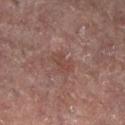Part of a total-body skin-imaging series; this lesion was reviewed on a skin check and was not flagged for biopsy. Located on the right leg. Imaged with cross-polarized lighting. The lesion-visualizer software estimated a classifier nevus-likeness of about 0/100. A 15 mm crop from a total-body photograph taken for skin-cancer surveillance. The patient is a female roughly 80 years of age. Measured at roughly 2.5 mm in maximum diameter.